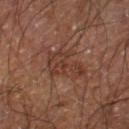Case summary:
- size · ~4.5 mm (longest diameter)
- image-analysis metrics · a lesion area of about 11 mm², an outline eccentricity of about 0.6 (0 = round, 1 = elongated), and two-axis asymmetry of about 0.45; a lesion–skin lightness drop of about 6 and a normalized border contrast of about 6; a detector confidence of about 100 out of 100 that the crop contains a lesion
- tile lighting · cross-polarized
- location · the right lower leg
- acquisition · ~15 mm crop, total-body skin-cancer survey
- patient · male, in their 60s